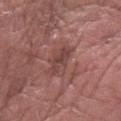This lesion was catalogued during total-body skin photography and was not selected for biopsy.
This is a white-light tile.
The subject is a male aged around 65.
The lesion-visualizer software estimated a footprint of about 5.5 mm², an eccentricity of roughly 0.9, and a symmetry-axis asymmetry near 0.3. It also reported about 8 CIELAB-L* units darker than the surrounding skin. The software also gave a border-irregularity rating of about 3.5/10.
The recorded lesion diameter is about 4 mm.
The lesion is located on the left forearm.
A 15 mm close-up extracted from a 3D total-body photography capture.